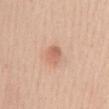Part of a total-body skin-imaging series; this lesion was reviewed on a skin check and was not flagged for biopsy. A region of skin cropped from a whole-body photographic capture, roughly 15 mm wide. The tile uses white-light illumination. Located on the mid back. Automated tile analysis of the lesion measured a border-irregularity index near 2/10, a within-lesion color-variation index near 3.5/10, and radial color variation of about 1.5. The software also gave a classifier nevus-likeness of about 65/100. A female subject, roughly 30 years of age. Approximately 3 mm at its widest.